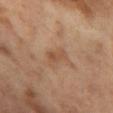{"lesion_size": {"long_diameter_mm_approx": 2.5}, "image": {"source": "total-body photography crop", "field_of_view_mm": 15}, "patient": {"sex": "female", "age_approx": 55}, "site": "left thigh"}